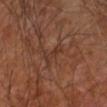{"biopsy_status": "not biopsied; imaged during a skin examination", "patient": {"sex": "male", "age_approx": 70}, "lesion_size": {"long_diameter_mm_approx": 2.5}, "automated_metrics": {"cielab_L": 33, "cielab_a": 19, "cielab_b": 26, "vs_skin_contrast_norm": 5.0, "border_irregularity_0_10": 4.5, "color_variation_0_10": 0.0}, "site": "right forearm", "lighting": "cross-polarized", "image": {"source": "total-body photography crop", "field_of_view_mm": 15}}